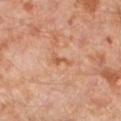biopsy_status: not biopsied; imaged during a skin examination
lighting: cross-polarized
patient:
  sex: male
  age_approx: 30
image:
  source: total-body photography crop
  field_of_view_mm: 15
site: leg